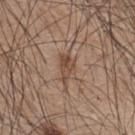A roughly 15 mm field-of-view crop from a total-body skin photograph. The lesion-visualizer software estimated a lesion color around L≈48 a*≈17 b*≈27 in CIELAB and a normalized border contrast of about 6.5. A male patient in their mid- to late 40s. Approximately 4 mm at its widest. Located on the upper back. This is a white-light tile.